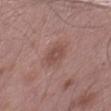No biopsy was performed on this lesion — it was imaged during a full skin examination and was not determined to be concerning. From the right thigh. A male patient approximately 50 years of age. Longest diameter approximately 3.5 mm. A 15 mm close-up extracted from a 3D total-body photography capture.